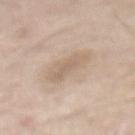Imaged during a routine full-body skin examination; the lesion was not biopsied and no histopathology is available.
The lesion is on the mid back.
The total-body-photography lesion software estimated an area of roughly 7 mm² and a symmetry-axis asymmetry near 0.25. The analysis additionally found a mean CIELAB color near L≈63 a*≈13 b*≈28 and a lesion–skin lightness drop of about 8. It also reported border irregularity of about 3.5 on a 0–10 scale and a within-lesion color-variation index near 2/10.
The subject is a male roughly 55 years of age.
Longest diameter approximately 4.5 mm.
Captured under white-light illumination.
A 15 mm close-up tile from a total-body photography series done for melanoma screening.